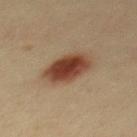The lesion was photographed on a routine skin check and not biopsied; there is no pathology result. Longest diameter approximately 5 mm. The patient is a male aged around 40. Cropped from a total-body skin-imaging series; the visible field is about 15 mm. The lesion-visualizer software estimated a mean CIELAB color near L≈37 a*≈19 b*≈27 and a lesion-to-skin contrast of about 11.5 (normalized; higher = more distinct). The software also gave a border-irregularity index near 1.5/10 and internal color variation of about 5 on a 0–10 scale. This is a cross-polarized tile. On the mid back.